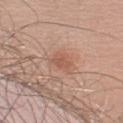Captured during whole-body skin photography for melanoma surveillance; the lesion was not biopsied.
The lesion's longest dimension is about 2.5 mm.
A roughly 15 mm field-of-view crop from a total-body skin photograph.
A male subject, aged approximately 60.
On the left upper arm.